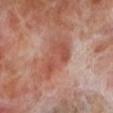{
  "biopsy_status": "not biopsied; imaged during a skin examination",
  "image": {
    "source": "total-body photography crop",
    "field_of_view_mm": 15
  },
  "patient": {
    "sex": "male",
    "age_approx": 70
  },
  "automated_metrics": {
    "border_irregularity_0_10": 4.0,
    "peripheral_color_asymmetry": 1.0,
    "nevus_likeness_0_100": 0,
    "lesion_detection_confidence_0_100": 100
  },
  "site": "left lower leg",
  "lesion_size": {
    "long_diameter_mm_approx": 5.5
  }
}